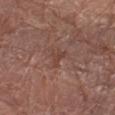Assessment:
No biopsy was performed on this lesion — it was imaged during a full skin examination and was not determined to be concerning.
Acquisition and patient details:
The lesion is on the right lower leg. The subject is a female approximately 80 years of age. Captured under white-light illumination. A 15 mm close-up tile from a total-body photography series done for melanoma screening. Longest diameter approximately 2.5 mm.